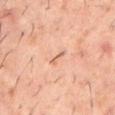{
  "biopsy_status": "not biopsied; imaged during a skin examination",
  "image": {
    "source": "total-body photography crop",
    "field_of_view_mm": 15
  },
  "lesion_size": {
    "long_diameter_mm_approx": 2.5
  },
  "site": "mid back",
  "patient": {
    "sex": "male",
    "age_approx": 60
  },
  "lighting": "cross-polarized"
}